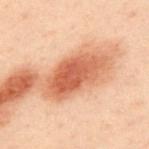follow-up = no biopsy performed (imaged during a skin exam)
imaging modality = 15 mm crop, total-body photography
automated lesion analysis = a lesion–skin lightness drop of about 13 and a normalized border contrast of about 9
subject = male, aged 48 to 52
body site = the upper back
lesion size = ≈7 mm